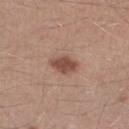{"biopsy_status": "not biopsied; imaged during a skin examination", "image": {"source": "total-body photography crop", "field_of_view_mm": 15}, "site": "right lower leg", "patient": {"sex": "male", "age_approx": 40}}